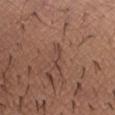{"biopsy_status": "not biopsied; imaged during a skin examination", "patient": {"sex": "male", "age_approx": 40}, "lesion_size": {"long_diameter_mm_approx": 3.5}, "site": "abdomen", "lighting": "white-light", "automated_metrics": {"cielab_L": 44, "cielab_a": 19, "cielab_b": 26, "vs_skin_darker_L": 7.0, "vs_skin_contrast_norm": 5.5, "border_irregularity_0_10": 7.5, "color_variation_0_10": 0.0, "peripheral_color_asymmetry": 0.0}, "image": {"source": "total-body photography crop", "field_of_view_mm": 15}}